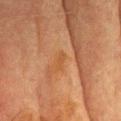Clinical impression: Imaged during a routine full-body skin examination; the lesion was not biopsied and no histopathology is available. Clinical summary: A male patient aged approximately 70. A 15 mm crop from a total-body photograph taken for skin-cancer surveillance. The lesion's longest dimension is about 2.5 mm. On the head or neck. The lesion-visualizer software estimated roughly 4 lightness units darker than nearby skin and a normalized lesion–skin contrast near 5. And it measured a border-irregularity index near 4.5/10, a color-variation rating of about 0/10, and a peripheral color-asymmetry measure near 0. It also reported a classifier nevus-likeness of about 0/100 and a detector confidence of about 80 out of 100 that the crop contains a lesion. This is a cross-polarized tile.